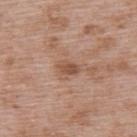Captured during whole-body skin photography for melanoma surveillance; the lesion was not biopsied. The tile uses white-light illumination. Measured at roughly 2.5 mm in maximum diameter. The patient is a male aged 48–52. A lesion tile, about 15 mm wide, cut from a 3D total-body photograph. The lesion is on the upper back.